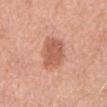Clinical impression: Part of a total-body skin-imaging series; this lesion was reviewed on a skin check and was not flagged for biopsy. Background: Imaged with white-light lighting. Cropped from a whole-body photographic skin survey; the tile spans about 15 mm. The lesion-visualizer software estimated an area of roughly 11 mm², an eccentricity of roughly 0.8, and a shape-asymmetry score of about 0.2 (0 = symmetric). The software also gave about 11 CIELAB-L* units darker than the surrounding skin and a normalized lesion–skin contrast near 7. The analysis additionally found a nevus-likeness score of about 85/100. The recorded lesion diameter is about 5 mm. The patient is a male aged around 70. From the mid back.